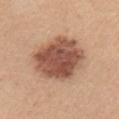Part of a total-body skin-imaging series; this lesion was reviewed on a skin check and was not flagged for biopsy. Located on the arm. A 15 mm close-up extracted from a 3D total-body photography capture. This is a white-light tile. The patient is a female in their mid-40s. Measured at roughly 7 mm in maximum diameter.